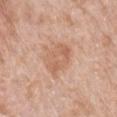Clinical impression:
The lesion was photographed on a routine skin check and not biopsied; there is no pathology result.
Context:
A female subject, aged approximately 70. From the left forearm. The lesion-visualizer software estimated a mean CIELAB color near L≈61 a*≈21 b*≈32 and a lesion-to-skin contrast of about 5.5 (normalized; higher = more distinct). The analysis additionally found a border-irregularity rating of about 2.5/10. The software also gave an automated nevus-likeness rating near 0 out of 100. This image is a 15 mm lesion crop taken from a total-body photograph. Imaged with white-light lighting.